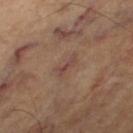Part of a total-body skin-imaging series; this lesion was reviewed on a skin check and was not flagged for biopsy. A subject aged 63–67. A lesion tile, about 15 mm wide, cut from a 3D total-body photograph. The lesion is on the right thigh.